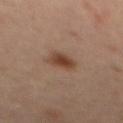A female patient in their mid-40s. Captured under cross-polarized illumination. From the mid back. About 3 mm across. A lesion tile, about 15 mm wide, cut from a 3D total-body photograph.